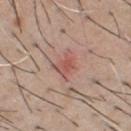<lesion>
<biopsy_status>not biopsied; imaged during a skin examination</biopsy_status>
<image>
  <source>total-body photography crop</source>
  <field_of_view_mm>15</field_of_view_mm>
</image>
<patient>
  <sex>male</sex>
  <age_approx>60</age_approx>
</patient>
<site>chest</site>
</lesion>